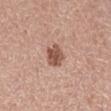{
  "biopsy_status": "not biopsied; imaged during a skin examination",
  "patient": {
    "sex": "female",
    "age_approx": 50
  },
  "image": {
    "source": "total-body photography crop",
    "field_of_view_mm": 15
  },
  "lesion_size": {
    "long_diameter_mm_approx": 2.5
  },
  "site": "left lower leg",
  "lighting": "white-light"
}